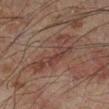{"biopsy_status": "not biopsied; imaged during a skin examination", "image": {"source": "total-body photography crop", "field_of_view_mm": 15}, "patient": {"sex": "male", "age_approx": 60}, "lesion_size": {"long_diameter_mm_approx": 6.5}, "site": "left leg"}